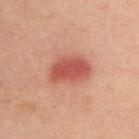Clinical impression:
The lesion was photographed on a routine skin check and not biopsied; there is no pathology result.
Clinical summary:
The lesion is located on the upper back. A male patient aged approximately 35. Automated tile analysis of the lesion measured an area of roughly 12 mm². And it measured an average lesion color of about L≈52 a*≈31 b*≈29 (CIELAB), roughly 12 lightness units darker than nearby skin, and a normalized lesion–skin contrast near 8. And it measured a border-irregularity rating of about 2/10, a color-variation rating of about 3.5/10, and peripheral color asymmetry of about 1. A lesion tile, about 15 mm wide, cut from a 3D total-body photograph.